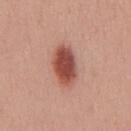Assessment: Imaged during a routine full-body skin examination; the lesion was not biopsied and no histopathology is available. Acquisition and patient details: Cropped from a total-body skin-imaging series; the visible field is about 15 mm. The lesion is on the chest. A male patient in their 30s.